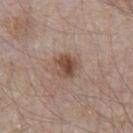follow-up: catalogued during a skin exam; not biopsied
automated lesion analysis: a shape-asymmetry score of about 0.25 (0 = symmetric); internal color variation of about 5 on a 0–10 scale and radial color variation of about 2
location: the front of the torso
imaging modality: total-body-photography crop, ~15 mm field of view
size: about 3 mm
patient: male, roughly 65 years of age
tile lighting: white-light